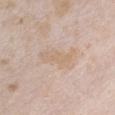Clinical impression:
This lesion was catalogued during total-body skin photography and was not selected for biopsy.
Acquisition and patient details:
This is a white-light tile. This image is a 15 mm lesion crop taken from a total-body photograph. From the chest. The subject is a female approximately 25 years of age. The lesion-visualizer software estimated an area of roughly 7 mm², an outline eccentricity of about 0.9 (0 = round, 1 = elongated), and a symmetry-axis asymmetry near 0.4. The analysis additionally found a border-irregularity index near 5/10, internal color variation of about 2 on a 0–10 scale, and a peripheral color-asymmetry measure near 1. The software also gave an automated nevus-likeness rating near 0 out of 100 and a lesion-detection confidence of about 100/100. Approximately 5 mm at its widest.